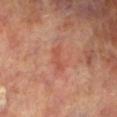Recorded during total-body skin imaging; not selected for excision or biopsy. The lesion is on the left lower leg. Cropped from a whole-body photographic skin survey; the tile spans about 15 mm. The subject is a male in their 70s. An algorithmic analysis of the crop reported a lesion area of about 3 mm², an eccentricity of roughly 0.95, and a shape-asymmetry score of about 0.3 (0 = symmetric).